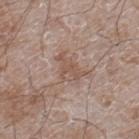Clinical impression:
Captured during whole-body skin photography for melanoma surveillance; the lesion was not biopsied.
Image and clinical context:
Located on the right lower leg. The patient is a male in their 60s. This image is a 15 mm lesion crop taken from a total-body photograph.